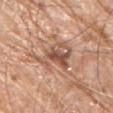The lesion was photographed on a routine skin check and not biopsied; there is no pathology result.
A 15 mm crop from a total-body photograph taken for skin-cancer surveillance.
A male subject aged 78 to 82.
The lesion is located on the arm.